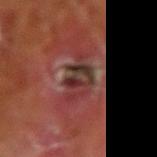Findings:
• lighting · cross-polarized illumination
• image · ~15 mm tile from a whole-body skin photo
• automated lesion analysis · a footprint of about 9 mm² and a symmetry-axis asymmetry near 0.35; an average lesion color of about L≈27 a*≈19 b*≈18 (CIELAB), about 7 CIELAB-L* units darker than the surrounding skin, and a normalized lesion–skin contrast near 7.5; a border-irregularity index near 6/10, internal color variation of about 9 on a 0–10 scale, and peripheral color asymmetry of about 4
• patient · male, in their 70s
• site · the right upper arm
• diameter · ≈4.5 mm